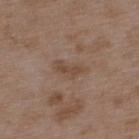The lesion was photographed on a routine skin check and not biopsied; there is no pathology result. A close-up tile cropped from a whole-body skin photograph, about 15 mm across. A male subject approximately 50 years of age. The lesion-visualizer software estimated a border-irregularity rating of about 5.5/10 and a peripheral color-asymmetry measure near 0. The lesion's longest dimension is about 3 mm. This is a white-light tile. Located on the upper back.